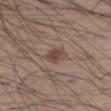workup=catalogued during a skin exam; not biopsied
size=≈3 mm
patient=male, aged 53 to 57
location=the right thigh
TBP lesion metrics=a border-irregularity index near 1.5/10 and a color-variation rating of about 4/10
image=total-body-photography crop, ~15 mm field of view